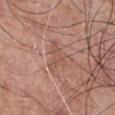follow-up = imaged on a skin check; not biopsied
subject = male, aged approximately 70
tile lighting = white-light illumination
automated lesion analysis = a lesion color around L≈53 a*≈22 b*≈27 in CIELAB and a normalized lesion–skin contrast near 5; border irregularity of about 6 on a 0–10 scale, a color-variation rating of about 2/10, and peripheral color asymmetry of about 1; a nevus-likeness score of about 0/100 and a detector confidence of about 100 out of 100 that the crop contains a lesion
body site = the chest
imaging modality = ~15 mm tile from a whole-body skin photo